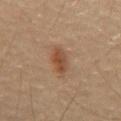follow-up=total-body-photography surveillance lesion; no biopsy
size=≈4 mm
tile lighting=cross-polarized illumination
imaging modality=~15 mm tile from a whole-body skin photo
patient=male, aged 53–57
site=the abdomen